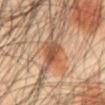Assessment: This lesion was catalogued during total-body skin photography and was not selected for biopsy. Context: The recorded lesion diameter is about 3 mm. A male patient, aged 43 to 47. Cropped from a total-body skin-imaging series; the visible field is about 15 mm. Located on the abdomen.